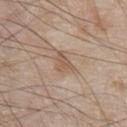Q: Was this lesion biopsied?
A: imaged on a skin check; not biopsied
Q: What is the anatomic site?
A: the chest
Q: Who is the patient?
A: male, aged around 80
Q: What kind of image is this?
A: 15 mm crop, total-body photography
Q: What did automated image analysis measure?
A: border irregularity of about 4 on a 0–10 scale, a color-variation rating of about 2/10, and peripheral color asymmetry of about 1
Q: Illumination type?
A: white-light